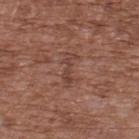Q: Was this lesion biopsied?
A: total-body-photography surveillance lesion; no biopsy
Q: What lighting was used for the tile?
A: white-light
Q: What did automated image analysis measure?
A: a footprint of about 3 mm², a shape eccentricity near 0.95, and two-axis asymmetry of about 0.45; a color-variation rating of about 0/10 and a peripheral color-asymmetry measure near 0
Q: What is the lesion's diameter?
A: ~3.5 mm (longest diameter)
Q: How was this image acquired?
A: ~15 mm crop, total-body skin-cancer survey
Q: Where on the body is the lesion?
A: the upper back
Q: What are the patient's age and sex?
A: male, roughly 75 years of age